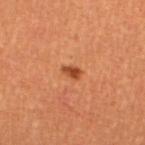Assessment:
Captured during whole-body skin photography for melanoma surveillance; the lesion was not biopsied.
Clinical summary:
A 15 mm close-up extracted from a 3D total-body photography capture. A female patient about 30 years old. On the left lower leg. This is a cross-polarized tile.